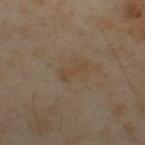Part of a total-body skin-imaging series; this lesion was reviewed on a skin check and was not flagged for biopsy. About 3 mm across. Located on the upper back. Cropped from a total-body skin-imaging series; the visible field is about 15 mm. Imaged with cross-polarized lighting. A male patient aged 53 to 57.